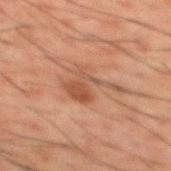Part of a total-body skin-imaging series; this lesion was reviewed on a skin check and was not flagged for biopsy.
Measured at roughly 5 mm in maximum diameter.
A 15 mm crop from a total-body photograph taken for skin-cancer surveillance.
From the mid back.
The subject is a male aged around 50.
The lesion-visualizer software estimated an area of roughly 9 mm², an outline eccentricity of about 0.75 (0 = round, 1 = elongated), and two-axis asymmetry of about 0.5. It also reported a border-irregularity rating of about 8.5/10, internal color variation of about 3 on a 0–10 scale, and peripheral color asymmetry of about 1. The software also gave an automated nevus-likeness rating near 0 out of 100.